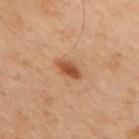biopsy status: total-body-photography surveillance lesion; no biopsy
patient: male, approximately 70 years of age
size: ~2.5 mm (longest diameter)
automated metrics: a footprint of about 4 mm², a shape eccentricity near 0.65, and two-axis asymmetry of about 0.15; a lesion-to-skin contrast of about 9 (normalized; higher = more distinct)
anatomic site: the arm
illumination: cross-polarized
imaging modality: ~15 mm tile from a whole-body skin photo